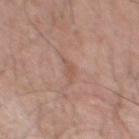Captured during whole-body skin photography for melanoma surveillance; the lesion was not biopsied.
Longest diameter approximately 2.5 mm.
The lesion is on the upper back.
Captured under white-light illumination.
A male subject, approximately 45 years of age.
A lesion tile, about 15 mm wide, cut from a 3D total-body photograph.